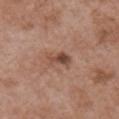Part of a total-body skin-imaging series; this lesion was reviewed on a skin check and was not flagged for biopsy. A female patient, aged around 75. From the chest. Cropped from a total-body skin-imaging series; the visible field is about 15 mm.